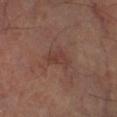Part of a total-body skin-imaging series; this lesion was reviewed on a skin check and was not flagged for biopsy. The lesion is on the right lower leg. The subject is approximately 65 years of age. Cropped from a total-body skin-imaging series; the visible field is about 15 mm.